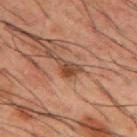Case summary:
* workup: total-body-photography surveillance lesion; no biopsy
* automated lesion analysis: a nevus-likeness score of about 70/100 and lesion-presence confidence of about 100/100
* illumination: cross-polarized
* body site: the mid back
* patient: male, in their 50s
* image: 15 mm crop, total-body photography